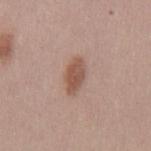The lesion was photographed on a routine skin check and not biopsied; there is no pathology result.
A 15 mm close-up tile from a total-body photography series done for melanoma screening.
Approximately 4 mm at its widest.
A male patient about 45 years old.
The lesion is located on the mid back.
Imaged with white-light lighting.